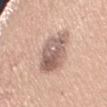Impression: Captured during whole-body skin photography for melanoma surveillance; the lesion was not biopsied. Background: The lesion is located on the right upper arm. This image is a 15 mm lesion crop taken from a total-body photograph. The tile uses white-light illumination. Measured at roughly 5 mm in maximum diameter. The patient is a female aged 28 to 32.